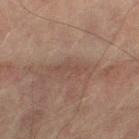The lesion was tiled from a total-body skin photograph and was not biopsied.
A male patient, aged 73 to 77.
On the right thigh.
Longest diameter approximately 3.5 mm.
Cropped from a total-body skin-imaging series; the visible field is about 15 mm.
Captured under cross-polarized illumination.
Automated tile analysis of the lesion measured an eccentricity of roughly 0.95 and a symmetry-axis asymmetry near 0.45.